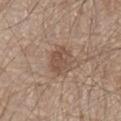{
  "biopsy_status": "not biopsied; imaged during a skin examination",
  "lighting": "white-light",
  "site": "chest",
  "patient": {
    "sex": "male",
    "age_approx": 80
  },
  "automated_metrics": {
    "cielab_L": 50,
    "cielab_a": 17,
    "cielab_b": 26,
    "vs_skin_darker_L": 9.0,
    "vs_skin_contrast_norm": 6.5,
    "border_irregularity_0_10": 2.5,
    "peripheral_color_asymmetry": 1.0
  },
  "image": {
    "source": "total-body photography crop",
    "field_of_view_mm": 15
  }
}